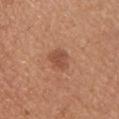Part of a total-body skin-imaging series; this lesion was reviewed on a skin check and was not flagged for biopsy.
Longest diameter approximately 3 mm.
From the left upper arm.
A 15 mm crop from a total-body photograph taken for skin-cancer surveillance.
This is a white-light tile.
A female patient roughly 25 years of age.